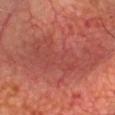Assessment:
Part of a total-body skin-imaging series; this lesion was reviewed on a skin check and was not flagged for biopsy.